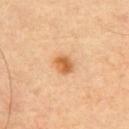* notes: catalogued during a skin exam; not biopsied